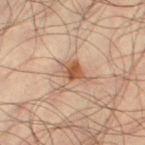follow-up: no biopsy performed (imaged during a skin exam)
lesion diameter: ≈4 mm
acquisition: total-body-photography crop, ~15 mm field of view
tile lighting: cross-polarized illumination
location: the leg
patient: male, roughly 35 years of age